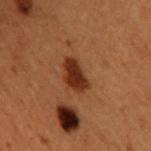Q: Is there a histopathology result?
A: catalogued during a skin exam; not biopsied
Q: What is the anatomic site?
A: the upper back
Q: What kind of image is this?
A: ~15 mm crop, total-body skin-cancer survey
Q: Lesion size?
A: about 3.5 mm
Q: Who is the patient?
A: male, aged 48 to 52
Q: What lighting was used for the tile?
A: cross-polarized illumination
Q: What did automated image analysis measure?
A: an area of roughly 7 mm², a shape eccentricity near 0.75, and two-axis asymmetry of about 0.25; a lesion color around L≈25 a*≈21 b*≈27 in CIELAB and about 11 CIELAB-L* units darker than the surrounding skin